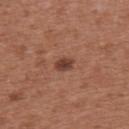follow-up — no biopsy performed (imaged during a skin exam)
imaging modality — ~15 mm crop, total-body skin-cancer survey
subject — female, about 40 years old
anatomic site — the upper back
lesion size — ≈2.5 mm
illumination — white-light illumination